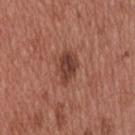The lesion was tiled from a total-body skin photograph and was not biopsied.